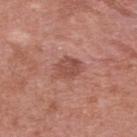notes = total-body-photography surveillance lesion; no biopsy
image = ~15 mm crop, total-body skin-cancer survey
size = about 3.5 mm
tile lighting = white-light illumination
body site = the upper back
subject = female, approximately 35 years of age